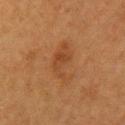Imaged during a routine full-body skin examination; the lesion was not biopsied and no histopathology is available. About 4.5 mm across. This is a cross-polarized tile. A 15 mm close-up extracted from a 3D total-body photography capture. Automated image analysis of the tile measured two-axis asymmetry of about 0.45. The analysis additionally found a mean CIELAB color near L≈36 a*≈20 b*≈31, roughly 6 lightness units darker than nearby skin, and a normalized lesion–skin contrast near 5.5. And it measured a border-irregularity index near 5/10, a color-variation rating of about 3/10, and radial color variation of about 1. And it measured an automated nevus-likeness rating near 10 out of 100 and a lesion-detection confidence of about 100/100. Located on the left upper arm. A female subject approximately 40 years of age.